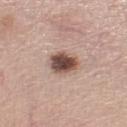Part of a total-body skin-imaging series; this lesion was reviewed on a skin check and was not flagged for biopsy. Cropped from a total-body skin-imaging series; the visible field is about 15 mm. The total-body-photography lesion software estimated lesion-presence confidence of about 100/100. On the left lower leg. Approximately 3.5 mm at its widest. The tile uses white-light illumination. The patient is a female in their mid-50s.